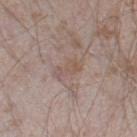Acquisition and patient details: A male subject, about 45 years old. A 15 mm close-up extracted from a 3D total-body photography capture. From the leg.